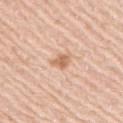Case summary:
– biopsy status · catalogued during a skin exam; not biopsied
– location · the right upper arm
– tile lighting · white-light
– image-analysis metrics · an area of roughly 3.5 mm², an outline eccentricity of about 0.7 (0 = round, 1 = elongated), and a symmetry-axis asymmetry near 0.3; an average lesion color of about L≈65 a*≈21 b*≈34 (CIELAB), a lesion–skin lightness drop of about 11, and a lesion-to-skin contrast of about 7 (normalized; higher = more distinct); internal color variation of about 2.5 on a 0–10 scale and radial color variation of about 1; a classifier nevus-likeness of about 75/100 and lesion-presence confidence of about 100/100
– imaging modality · ~15 mm tile from a whole-body skin photo
– lesion size · ≈2.5 mm
– patient · male, in their mid- to late 60s